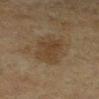{
  "biopsy_status": "not biopsied; imaged during a skin examination",
  "image": {
    "source": "total-body photography crop",
    "field_of_view_mm": 15
  },
  "site": "leg",
  "patient": {
    "sex": "female",
    "age_approx": 55
  }
}